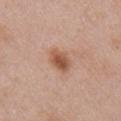Assessment:
No biopsy was performed on this lesion — it was imaged during a full skin examination and was not determined to be concerning.
Image and clinical context:
Approximately 3.5 mm at its widest. A 15 mm crop from a total-body photograph taken for skin-cancer surveillance. Located on the right upper arm. A female patient, in their 50s.